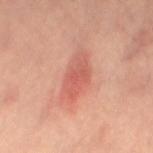| key | value |
|---|---|
| biopsy status | catalogued during a skin exam; not biopsied |
| patient | female, about 40 years old |
| acquisition | total-body-photography crop, ~15 mm field of view |
| location | the right leg |